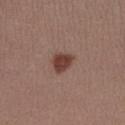Case summary:
* follow-up — imaged on a skin check; not biopsied
* body site — the right forearm
* diameter — ~3 mm (longest diameter)
* image — 15 mm crop, total-body photography
* TBP lesion metrics — a lesion color around L≈40 a*≈21 b*≈24 in CIELAB, a lesion–skin lightness drop of about 12, and a lesion-to-skin contrast of about 10 (normalized; higher = more distinct); a classifier nevus-likeness of about 100/100
* patient — female, about 30 years old